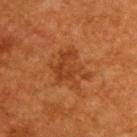Imaged during a routine full-body skin examination; the lesion was not biopsied and no histopathology is available.
Measured at roughly 5 mm in maximum diameter.
On the back.
The patient is a male aged 58 to 62.
Cropped from a total-body skin-imaging series; the visible field is about 15 mm.
Captured under cross-polarized illumination.